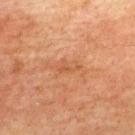No biopsy was performed on this lesion — it was imaged during a full skin examination and was not determined to be concerning. Imaged with cross-polarized lighting. A 15 mm close-up extracted from a 3D total-body photography capture. On the upper back. The patient is a male aged approximately 75.